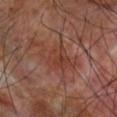acquisition: 15 mm crop, total-body photography; illumination: cross-polarized illumination; body site: the right forearm; patient: male, aged around 70.